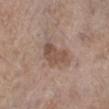notes=total-body-photography surveillance lesion; no biopsy | lighting=white-light illumination | patient=female, roughly 85 years of age or older | site=the right lower leg | imaging modality=~15 mm tile from a whole-body skin photo | size=about 4.5 mm.